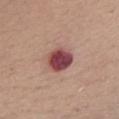Q: What is the imaging modality?
A: 15 mm crop, total-body photography
Q: Lesion size?
A: ~3.5 mm (longest diameter)
Q: Where on the body is the lesion?
A: the chest
Q: What are the patient's age and sex?
A: female, aged 63–67